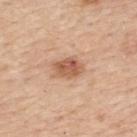This lesion was catalogued during total-body skin photography and was not selected for biopsy.
A close-up tile cropped from a whole-body skin photograph, about 15 mm across.
A female subject in their mid-60s.
The tile uses white-light illumination.
Approximately 3.5 mm at its widest.
Located on the back.